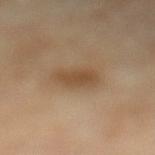Assessment: The lesion was tiled from a total-body skin photograph and was not biopsied. Context: The total-body-photography lesion software estimated a lesion color around L≈49 a*≈17 b*≈33 in CIELAB and a normalized lesion–skin contrast near 7. And it measured a border-irregularity index near 2.5/10, internal color variation of about 2 on a 0–10 scale, and peripheral color asymmetry of about 0.5. The patient is a female roughly 70 years of age. Measured at roughly 3.5 mm in maximum diameter. From the left lower leg. A region of skin cropped from a whole-body photographic capture, roughly 15 mm wide. The tile uses cross-polarized illumination.